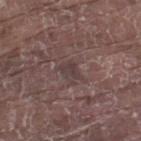workup=total-body-photography surveillance lesion; no biopsy
lesion diameter=~2.5 mm (longest diameter)
patient=male, roughly 80 years of age
site=the right lower leg
lighting=white-light illumination
imaging modality=~15 mm crop, total-body skin-cancer survey